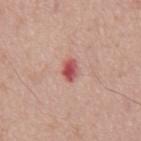acquisition: total-body-photography crop, ~15 mm field of view | anatomic site: the mid back | automated metrics: a shape-asymmetry score of about 0.25 (0 = symmetric); a border-irregularity index near 2.5/10 and radial color variation of about 1; an automated nevus-likeness rating near 40 out of 100 and a detector confidence of about 100 out of 100 that the crop contains a lesion | patient: male, aged approximately 65.